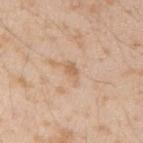<record>
  <biopsy_status>not biopsied; imaged during a skin examination</biopsy_status>
  <image>
    <source>total-body photography crop</source>
    <field_of_view_mm>15</field_of_view_mm>
  </image>
  <site>arm</site>
  <lighting>white-light</lighting>
  <lesion_size>
    <long_diameter_mm_approx>3.0</long_diameter_mm_approx>
  </lesion_size>
  <patient>
    <sex>male</sex>
    <age_approx>25</age_approx>
  </patient>
  <automated_metrics>
    <cielab_L>63</cielab_L>
    <cielab_a>18</cielab_a>
    <cielab_b>33</cielab_b>
    <vs_skin_darker_L>8.0</vs_skin_darker_L>
    <vs_skin_contrast_norm>5.5</vs_skin_contrast_norm>
  </automated_metrics>
</record>